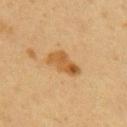<tbp_lesion>
  <biopsy_status>not biopsied; imaged during a skin examination</biopsy_status>
  <site>right upper arm</site>
  <lighting>cross-polarized</lighting>
  <patient>
    <sex>female</sex>
    <age_approx>30</age_approx>
  </patient>
  <image>
    <source>total-body photography crop</source>
    <field_of_view_mm>15</field_of_view_mm>
  </image>
</tbp_lesion>